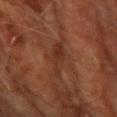{"biopsy_status": "not biopsied; imaged during a skin examination", "lesion_size": {"long_diameter_mm_approx": 5.5}, "patient": {"sex": "male", "age_approx": 70}, "image": {"source": "total-body photography crop", "field_of_view_mm": 15}, "automated_metrics": {"area_mm2_approx": 6.5, "border_irregularity_0_10": 4.5, "color_variation_0_10": 2.0, "peripheral_color_asymmetry": 0.5}, "site": "right upper arm", "lighting": "cross-polarized"}